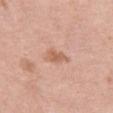Clinical impression:
The lesion was tiled from a total-body skin photograph and was not biopsied.
Background:
Automated image analysis of the tile measured a normalized border contrast of about 7. Imaged with white-light lighting. The recorded lesion diameter is about 2.5 mm. Cropped from a total-body skin-imaging series; the visible field is about 15 mm. A female subject aged around 40. The lesion is located on the left thigh.